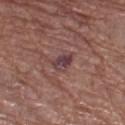| field | value |
|---|---|
| acquisition | ~15 mm crop, total-body skin-cancer survey |
| patient | female, in their mid-70s |
| tile lighting | white-light |
| body site | the left thigh |